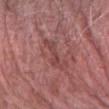The lesion was photographed on a routine skin check and not biopsied; there is no pathology result.
The total-body-photography lesion software estimated a border-irregularity index near 8.5/10 and a color-variation rating of about 1.5/10.
The patient is a male aged around 80.
This image is a 15 mm lesion crop taken from a total-body photograph.
Approximately 5 mm at its widest.
The lesion is on the arm.
The tile uses white-light illumination.